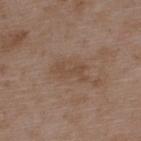notes = no biopsy performed (imaged during a skin exam); image source = total-body-photography crop, ~15 mm field of view; patient = male, aged approximately 50; location = the upper back.